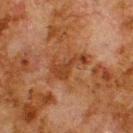No biopsy was performed on this lesion — it was imaged during a full skin examination and was not determined to be concerning.
Located on the upper back.
This image is a 15 mm lesion crop taken from a total-body photograph.
A male subject, aged 78 to 82.